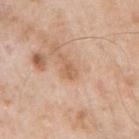Assessment:
Part of a total-body skin-imaging series; this lesion was reviewed on a skin check and was not flagged for biopsy.
Context:
Longest diameter approximately 2.5 mm. From the left upper arm. A 15 mm crop from a total-body photograph taken for skin-cancer surveillance. A male patient aged around 55. The tile uses white-light illumination.